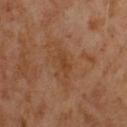The lesion was tiled from a total-body skin photograph and was not biopsied. Automated tile analysis of the lesion measured a lesion area of about 3 mm², an outline eccentricity of about 0.8 (0 = round, 1 = elongated), and a symmetry-axis asymmetry near 0.25. The analysis additionally found an average lesion color of about L≈40 a*≈21 b*≈33 (CIELAB). The analysis additionally found a border-irregularity rating of about 2/10, a color-variation rating of about 1.5/10, and radial color variation of about 0.5. A male subject, approximately 65 years of age. Cropped from a total-body skin-imaging series; the visible field is about 15 mm. This is a cross-polarized tile.